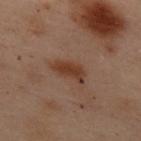{"biopsy_status": "not biopsied; imaged during a skin examination", "site": "upper back", "lighting": "cross-polarized", "patient": {"sex": "female", "age_approx": 55}, "image": {"source": "total-body photography crop", "field_of_view_mm": 15}, "automated_metrics": {"cielab_L": 30, "cielab_a": 17, "cielab_b": 24, "vs_skin_darker_L": 8.0, "vs_skin_contrast_norm": 8.5}, "lesion_size": {"long_diameter_mm_approx": 4.0}}